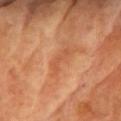No biopsy was performed on this lesion — it was imaged during a full skin examination and was not determined to be concerning. A female patient approximately 75 years of age. The lesion is located on the upper back. Cropped from a total-body skin-imaging series; the visible field is about 15 mm.